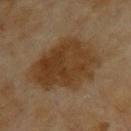No biopsy was performed on this lesion — it was imaged during a full skin examination and was not determined to be concerning.
The lesion is on the back.
An algorithmic analysis of the crop reported an average lesion color of about L≈34 a*≈15 b*≈30 (CIELAB) and a normalized border contrast of about 9. The software also gave a border-irregularity rating of about 3/10 and peripheral color asymmetry of about 1.5. The software also gave a classifier nevus-likeness of about 95/100 and a lesion-detection confidence of about 100/100.
A female subject, aged approximately 60.
Cropped from a total-body skin-imaging series; the visible field is about 15 mm.
The tile uses cross-polarized illumination.